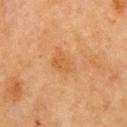Q: Was this lesion biopsied?
A: no biopsy performed (imaged during a skin exam)
Q: Illumination type?
A: cross-polarized illumination
Q: What is the anatomic site?
A: the arm
Q: Who is the patient?
A: female, approximately 70 years of age
Q: Lesion size?
A: ~3.5 mm (longest diameter)
Q: What kind of image is this?
A: ~15 mm tile from a whole-body skin photo